The lesion is located on the left thigh; cropped from a whole-body photographic skin survey; the tile spans about 15 mm; the patient is a male about 60 years old:
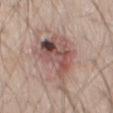Notes:
• histopathology — a squamous cell carcinoma in situ